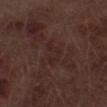biopsy status = total-body-photography surveillance lesion; no biopsy
diameter = ≈3.5 mm
imaging modality = ~15 mm tile from a whole-body skin photo
tile lighting = white-light illumination
location = the right thigh
patient = male, in their 70s